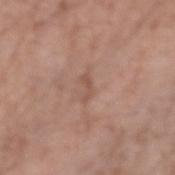Impression: Captured during whole-body skin photography for melanoma surveillance; the lesion was not biopsied. Acquisition and patient details: A male patient aged 68–72. From the right forearm. A region of skin cropped from a whole-body photographic capture, roughly 15 mm wide.